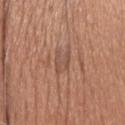Part of a total-body skin-imaging series; this lesion was reviewed on a skin check and was not flagged for biopsy.
The total-body-photography lesion software estimated an area of roughly 4 mm², an outline eccentricity of about 0.75 (0 = round, 1 = elongated), and a shape-asymmetry score of about 0.45 (0 = symmetric). It also reported a classifier nevus-likeness of about 0/100 and a lesion-detection confidence of about 60/100.
The lesion is on the head or neck.
A male subject about 60 years old.
This image is a 15 mm lesion crop taken from a total-body photograph.